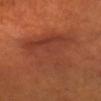This lesion was catalogued during total-body skin photography and was not selected for biopsy.
The lesion is located on the head or neck.
A close-up tile cropped from a whole-body skin photograph, about 15 mm across.
The tile uses cross-polarized illumination.
A male subject, in their 60s.
Approximately 8 mm at its widest.